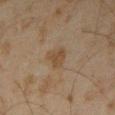Impression: Part of a total-body skin-imaging series; this lesion was reviewed on a skin check and was not flagged for biopsy. Context: This image is a 15 mm lesion crop taken from a total-body photograph. From the left forearm. The lesion-visualizer software estimated border irregularity of about 3.5 on a 0–10 scale and internal color variation of about 1.5 on a 0–10 scale. This is a cross-polarized tile. A male patient approximately 45 years of age. About 3 mm across.